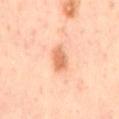The lesion was photographed on a routine skin check and not biopsied; there is no pathology result. The patient is a male in their mid- to late 60s. The tile uses cross-polarized illumination. The lesion's longest dimension is about 3.5 mm. The lesion is on the mid back. This image is a 15 mm lesion crop taken from a total-body photograph.